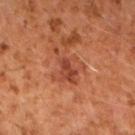notes = catalogued during a skin exam; not biopsied
image = total-body-photography crop, ~15 mm field of view
subject = male, aged approximately 60
illumination = cross-polarized
location = the right forearm
lesion diameter = about 4.5 mm